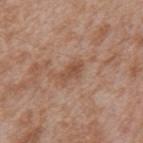| field | value |
|---|---|
| notes | imaged on a skin check; not biopsied |
| illumination | white-light illumination |
| image-analysis metrics | a border-irregularity index near 3.5/10 and a color-variation rating of about 1.5/10 |
| patient | male, aged around 65 |
| location | the mid back |
| lesion size | ~3 mm (longest diameter) |
| image source | 15 mm crop, total-body photography |